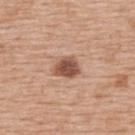  biopsy_status: not biopsied; imaged during a skin examination
  site: upper back
  image:
    source: total-body photography crop
    field_of_view_mm: 15
  patient:
    sex: female
    age_approx: 65